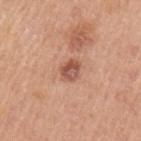{"biopsy_status": "not biopsied; imaged during a skin examination", "image": {"source": "total-body photography crop", "field_of_view_mm": 15}, "site": "arm", "lesion_size": {"long_diameter_mm_approx": 3.0}, "patient": {"sex": "male", "age_approx": 50}, "lighting": "white-light"}